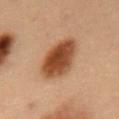Impression:
The lesion was tiled from a total-body skin photograph and was not biopsied.
Clinical summary:
The subject is a male about 55 years old. The lesion is on the abdomen. Cropped from a total-body skin-imaging series; the visible field is about 15 mm.